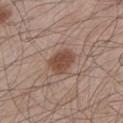workup = catalogued during a skin exam; not biopsied.